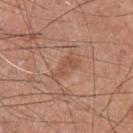Assessment:
Captured during whole-body skin photography for melanoma surveillance; the lesion was not biopsied.
Acquisition and patient details:
Imaged with white-light lighting. Cropped from a whole-body photographic skin survey; the tile spans about 15 mm. The recorded lesion diameter is about 3.5 mm. The lesion is located on the front of the torso. A male patient roughly 55 years of age.